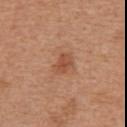| key | value |
|---|---|
| location | the abdomen |
| subject | male, about 65 years old |
| imaging modality | ~15 mm crop, total-body skin-cancer survey |
| illumination | white-light |
| size | ≈3 mm |